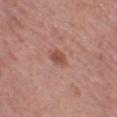Q: Is there a histopathology result?
A: catalogued during a skin exam; not biopsied
Q: What did automated image analysis measure?
A: an area of roughly 3.5 mm² and a shape eccentricity near 0.85; a mean CIELAB color near L≈50 a*≈24 b*≈27, roughly 10 lightness units darker than nearby skin, and a normalized border contrast of about 7.5; a border-irregularity index near 2.5/10 and a peripheral color-asymmetry measure near 0.5; an automated nevus-likeness rating near 75 out of 100
Q: What lighting was used for the tile?
A: white-light
Q: What kind of image is this?
A: ~15 mm tile from a whole-body skin photo
Q: Who is the patient?
A: female, aged 48–52
Q: Where on the body is the lesion?
A: the left thigh